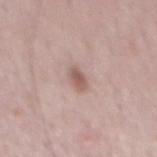Captured during whole-body skin photography for melanoma surveillance; the lesion was not biopsied. From the mid back. A male subject in their 50s. About 2.5 mm across. A region of skin cropped from a whole-body photographic capture, roughly 15 mm wide. This is a white-light tile.